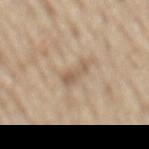  biopsy_status: not biopsied; imaged during a skin examination
  lighting: white-light
  automated_metrics:
    nevus_likeness_0_100: 10
    lesion_detection_confidence_0_100: 100
  patient:
    sex: male
    age_approx: 70
  image:
    source: total-body photography crop
    field_of_view_mm: 15
  site: mid back
  lesion_size:
    long_diameter_mm_approx: 2.5